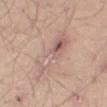Clinical impression:
The lesion was photographed on a routine skin check and not biopsied; there is no pathology result.
Acquisition and patient details:
Approximately 8.5 mm at its widest. A male subject in their mid-20s. The lesion is on the right thigh. A 15 mm close-up extracted from a 3D total-body photography capture. This is a white-light tile. An algorithmic analysis of the crop reported a nevus-likeness score of about 0/100.